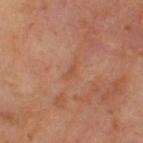{
  "biopsy_status": "not biopsied; imaged during a skin examination",
  "image": {
    "source": "total-body photography crop",
    "field_of_view_mm": 15
  },
  "patient": {
    "sex": "male",
    "age_approx": 65
  },
  "lesion_size": {
    "long_diameter_mm_approx": 2.5
  },
  "automated_metrics": {
    "cielab_L": 50,
    "cielab_a": 23,
    "cielab_b": 33,
    "vs_skin_contrast_norm": 5.0,
    "border_irregularity_0_10": 5.0,
    "color_variation_0_10": 0.0
  },
  "lighting": "cross-polarized"
}